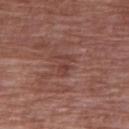Approximately 2.5 mm at its widest. From the right upper arm. A female subject, about 70 years old. The tile uses white-light illumination. A close-up tile cropped from a whole-body skin photograph, about 15 mm across.